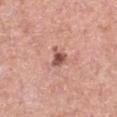No biopsy was performed on this lesion — it was imaged during a full skin examination and was not determined to be concerning. From the arm. Cropped from a total-body skin-imaging series; the visible field is about 15 mm. A female patient aged 38 to 42. The tile uses white-light illumination.